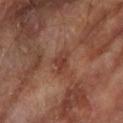Imaged during a routine full-body skin examination; the lesion was not biopsied and no histopathology is available. The lesion-visualizer software estimated an area of roughly 3.5 mm² and a symmetry-axis asymmetry near 0.4. The analysis additionally found a border-irregularity index near 4/10 and a color-variation rating of about 2.5/10. A lesion tile, about 15 mm wide, cut from a 3D total-body photograph. A male subject, in their mid-80s or older. About 2.5 mm across. Captured under cross-polarized illumination. On the left forearm.